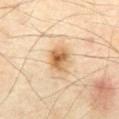Part of a total-body skin-imaging series; this lesion was reviewed on a skin check and was not flagged for biopsy. Cropped from a total-body skin-imaging series; the visible field is about 15 mm. The patient is a male aged 68–72. The lesion is located on the front of the torso. About 3.5 mm across. This is a cross-polarized tile.